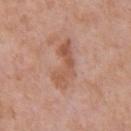biopsy_status: not biopsied; imaged during a skin examination
image:
  source: total-body photography crop
  field_of_view_mm: 15
automated_metrics:
  area_mm2_approx: 9.5
  eccentricity: 0.95
  shape_asymmetry: 0.45
patient:
  sex: male
  age_approx: 70
lighting: white-light
site: chest